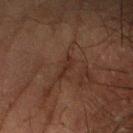workup: catalogued during a skin exam; not biopsied.